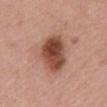Findings:
– follow-up: imaged on a skin check; not biopsied
– image source: 15 mm crop, total-body photography
– patient: female, aged 68–72
– image-analysis metrics: a lesion area of about 16 mm² and a shape-asymmetry score of about 0.1 (0 = symmetric); a mean CIELAB color near L≈48 a*≈24 b*≈29 and a lesion–skin lightness drop of about 16; a border-irregularity index near 1.5/10, a color-variation rating of about 7.5/10, and a peripheral color-asymmetry measure near 2.5; a classifier nevus-likeness of about 95/100 and a lesion-detection confidence of about 100/100
– anatomic site: the back
– diameter: about 6 mm